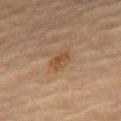workup = total-body-photography surveillance lesion; no biopsy | imaging modality = ~15 mm crop, total-body skin-cancer survey | patient = male, roughly 85 years of age or older | location = the mid back | illumination = cross-polarized illumination | TBP lesion metrics = border irregularity of about 3 on a 0–10 scale and peripheral color asymmetry of about 1 | diameter = ≈2.5 mm.